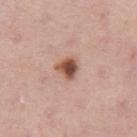biopsy status=no biopsy performed (imaged during a skin exam)
TBP lesion metrics=a lesion–skin lightness drop of about 16
location=the leg
image source=15 mm crop, total-body photography
subject=male, aged 73–77